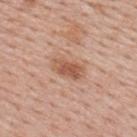The lesion was photographed on a routine skin check and not biopsied; there is no pathology result.
The lesion-visualizer software estimated a lesion area of about 6 mm². The software also gave a mean CIELAB color near L≈55 a*≈23 b*≈31. The analysis additionally found an automated nevus-likeness rating near 60 out of 100 and a lesion-detection confidence of about 100/100.
The recorded lesion diameter is about 3.5 mm.
A 15 mm close-up extracted from a 3D total-body photography capture.
This is a white-light tile.
A male subject, aged around 40.
From the upper back.